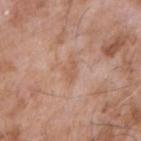No biopsy was performed on this lesion — it was imaged during a full skin examination and was not determined to be concerning. The lesion's longest dimension is about 2.5 mm. Cropped from a total-body skin-imaging series; the visible field is about 15 mm. The subject is a male aged around 75. From the right forearm.